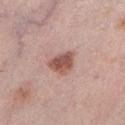Findings:
• lesion size — about 3.5 mm
• patient — female, in their mid- to late 60s
• illumination — white-light illumination
• body site — the left lower leg
• image-analysis metrics — an average lesion color of about L≈53 a*≈23 b*≈25 (CIELAB), about 13 CIELAB-L* units darker than the surrounding skin, and a normalized border contrast of about 9; a classifier nevus-likeness of about 90/100 and lesion-presence confidence of about 100/100
• image source — 15 mm crop, total-body photography